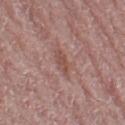The lesion was tiled from a total-body skin photograph and was not biopsied. From the leg. Cropped from a whole-body photographic skin survey; the tile spans about 15 mm. A female patient aged around 65. Longest diameter approximately 3 mm. This is a white-light tile.